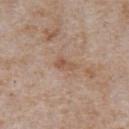Recorded during total-body skin imaging; not selected for excision or biopsy. A 15 mm close-up tile from a total-body photography series done for melanoma screening. About 2.5 mm across. The lesion is on the chest. The lesion-visualizer software estimated a mean CIELAB color near L≈54 a*≈19 b*≈29, a lesion–skin lightness drop of about 8, and a lesion-to-skin contrast of about 6 (normalized; higher = more distinct). The subject is a male about 65 years old. Imaged with white-light lighting.